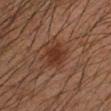Imaged during a routine full-body skin examination; the lesion was not biopsied and no histopathology is available. A male subject, approximately 35 years of age. A lesion tile, about 15 mm wide, cut from a 3D total-body photograph. The lesion's longest dimension is about 3.5 mm. The tile uses cross-polarized illumination. On the left forearm.